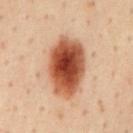Notes:
* biopsy status — imaged on a skin check; not biopsied
* tile lighting — cross-polarized
* body site — the mid back
* lesion size — ≈7.5 mm
* image — ~15 mm crop, total-body skin-cancer survey
* patient — male, aged approximately 45